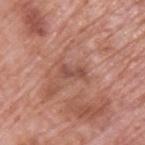No biopsy was performed on this lesion — it was imaged during a full skin examination and was not determined to be concerning.
This image is a 15 mm lesion crop taken from a total-body photograph.
The tile uses white-light illumination.
From the back.
A male subject aged 68–72.